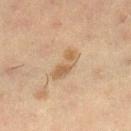Captured during whole-body skin photography for melanoma surveillance; the lesion was not biopsied. From the right lower leg. A female subject, aged approximately 40. This image is a 15 mm lesion crop taken from a total-body photograph.